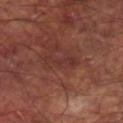Assessment:
Part of a total-body skin-imaging series; this lesion was reviewed on a skin check and was not flagged for biopsy.
Image and clinical context:
About 4 mm across. The tile uses cross-polarized illumination. From the right lower leg. A lesion tile, about 15 mm wide, cut from a 3D total-body photograph. The subject is a male aged approximately 60.